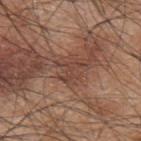Assessment: Recorded during total-body skin imaging; not selected for excision or biopsy. Image and clinical context: The recorded lesion diameter is about 3 mm. The lesion is located on the upper back. The subject is a male aged 43–47. A region of skin cropped from a whole-body photographic capture, roughly 15 mm wide. The lesion-visualizer software estimated a lesion-detection confidence of about 75/100. This is a white-light tile.